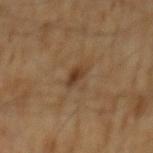Q: Was this lesion biopsied?
A: catalogued during a skin exam; not biopsied
Q: What lighting was used for the tile?
A: cross-polarized
Q: What is the anatomic site?
A: the mid back
Q: Lesion size?
A: ≈2.5 mm
Q: Automated lesion metrics?
A: a lesion color around L≈35 a*≈16 b*≈28 in CIELAB, a lesion–skin lightness drop of about 8, and a normalized lesion–skin contrast near 7.5; a border-irregularity index near 2.5/10 and a within-lesion color-variation index near 3/10
Q: How was this image acquired?
A: ~15 mm tile from a whole-body skin photo
Q: Patient demographics?
A: male, in their 60s